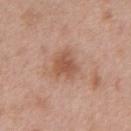<lesion>
  <biopsy_status>not biopsied; imaged during a skin examination</biopsy_status>
  <site>abdomen</site>
  <patient>
    <sex>male</sex>
    <age_approx>50</age_approx>
  </patient>
  <lesion_size>
    <long_diameter_mm_approx>3.5</long_diameter_mm_approx>
  </lesion_size>
  <image>
    <source>total-body photography crop</source>
    <field_of_view_mm>15</field_of_view_mm>
  </image>
</lesion>